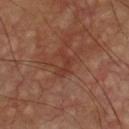Part of a total-body skin-imaging series; this lesion was reviewed on a skin check and was not flagged for biopsy.
Automated image analysis of the tile measured an average lesion color of about L≈36 a*≈24 b*≈27 (CIELAB), a lesion–skin lightness drop of about 6, and a normalized lesion–skin contrast near 5.5. It also reported an automated nevus-likeness rating near 0 out of 100 and a detector confidence of about 95 out of 100 that the crop contains a lesion.
The lesion is located on the front of the torso.
Cropped from a whole-body photographic skin survey; the tile spans about 15 mm.
A male subject, aged 58–62.
This is a cross-polarized tile.